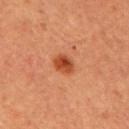Q: Was a biopsy performed?
A: imaged on a skin check; not biopsied
Q: What is the anatomic site?
A: the chest
Q: Who is the patient?
A: male, aged approximately 65
Q: How large is the lesion?
A: ≈2.5 mm
Q: How was the tile lit?
A: cross-polarized
Q: What is the imaging modality?
A: 15 mm crop, total-body photography
Q: Automated lesion metrics?
A: a mean CIELAB color near L≈44 a*≈30 b*≈37, roughly 12 lightness units darker than nearby skin, and a normalized border contrast of about 9.5; a within-lesion color-variation index near 4/10 and peripheral color asymmetry of about 1.5; a lesion-detection confidence of about 100/100